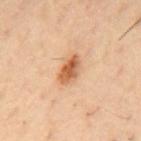Captured during whole-body skin photography for melanoma surveillance; the lesion was not biopsied. This image is a 15 mm lesion crop taken from a total-body photograph. The lesion is located on the mid back. Captured under cross-polarized illumination. The subject is a male about 55 years old. Automated tile analysis of the lesion measured a shape eccentricity near 0.8 and a symmetry-axis asymmetry near 0.2. It also reported a mean CIELAB color near L≈59 a*≈23 b*≈37, about 13 CIELAB-L* units darker than the surrounding skin, and a normalized border contrast of about 9.